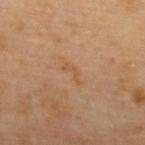Case summary:
* follow-up: catalogued during a skin exam; not biopsied
* imaging modality: ~15 mm tile from a whole-body skin photo
* illumination: cross-polarized illumination
* patient: female, aged around 65
* site: the upper back
* size: ~3 mm (longest diameter)